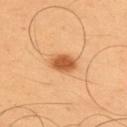<tbp_lesion>
<biopsy_status>not biopsied; imaged during a skin examination</biopsy_status>
<lighting>cross-polarized</lighting>
<site>back</site>
<image>
  <source>total-body photography crop</source>
  <field_of_view_mm>15</field_of_view_mm>
</image>
<lesion_size>
  <long_diameter_mm_approx>3.0</long_diameter_mm_approx>
</lesion_size>
<patient>
  <sex>male</sex>
  <age_approx>55</age_approx>
</patient>
</tbp_lesion>